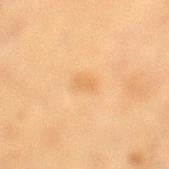<record>
  <image>
    <source>total-body photography crop</source>
    <field_of_view_mm>15</field_of_view_mm>
  </image>
  <site>left lower leg</site>
  <patient>
    <sex>female</sex>
    <age_approx>55</age_approx>
  </patient>
</record>